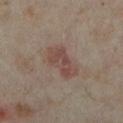From the left lower leg. This image is a 15 mm lesion crop taken from a total-body photograph. The patient is a female approximately 35 years of age.